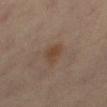Impression: The lesion was tiled from a total-body skin photograph and was not biopsied. Clinical summary: A male patient aged around 60. This image is a 15 mm lesion crop taken from a total-body photograph. From the right thigh.